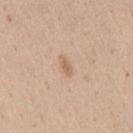Imaged during a routine full-body skin examination; the lesion was not biopsied and no histopathology is available. The tile uses white-light illumination. On the mid back. A female subject, roughly 40 years of age. A 15 mm close-up extracted from a 3D total-body photography capture. The lesion's longest dimension is about 2.5 mm.